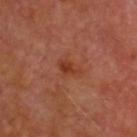Clinical impression: Imaged during a routine full-body skin examination; the lesion was not biopsied and no histopathology is available. Image and clinical context: The lesion is on the head or neck. About 3 mm across. The tile uses cross-polarized illumination. Automated image analysis of the tile measured a footprint of about 4 mm² and a shape eccentricity near 0.8. And it measured an average lesion color of about L≈37 a*≈27 b*≈32 (CIELAB), about 7 CIELAB-L* units darker than the surrounding skin, and a normalized border contrast of about 7. The analysis additionally found a border-irregularity rating of about 3.5/10 and internal color variation of about 2 on a 0–10 scale. The software also gave a classifier nevus-likeness of about 20/100 and a detector confidence of about 100 out of 100 that the crop contains a lesion. A 15 mm close-up tile from a total-body photography series done for melanoma screening. A male subject, in their 60s.